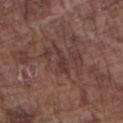No biopsy was performed on this lesion — it was imaged during a full skin examination and was not determined to be concerning.